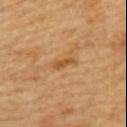{
  "biopsy_status": "not biopsied; imaged during a skin examination"
}